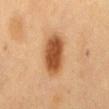biopsy status = no biopsy performed (imaged during a skin exam)
location = the mid back
image source = 15 mm crop, total-body photography
automated lesion analysis = a mean CIELAB color near L≈53 a*≈24 b*≈39, roughly 16 lightness units darker than nearby skin, and a normalized lesion–skin contrast near 10.5; an automated nevus-likeness rating near 100 out of 100 and a detector confidence of about 100 out of 100 that the crop contains a lesion
lesion diameter = about 6 mm
subject = male, aged approximately 60
tile lighting = cross-polarized